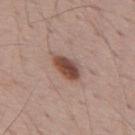Findings:
- follow-up — catalogued during a skin exam; not biopsied
- image — ~15 mm crop, total-body skin-cancer survey
- body site — the mid back
- subject — male, in their 70s